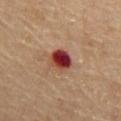biopsy status = no biopsy performed (imaged during a skin exam) | subject = male, aged 83 or over | anatomic site = the chest | image source = ~15 mm crop, total-body skin-cancer survey | size = about 3 mm.